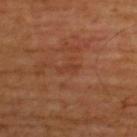Clinical impression:
Imaged during a routine full-body skin examination; the lesion was not biopsied and no histopathology is available.
Image and clinical context:
A male subject aged 63–67. On the upper back. The lesion's longest dimension is about 2.5 mm. Automated image analysis of the tile measured border irregularity of about 4.5 on a 0–10 scale, internal color variation of about 0 on a 0–10 scale, and radial color variation of about 0. The analysis additionally found a detector confidence of about 100 out of 100 that the crop contains a lesion. A lesion tile, about 15 mm wide, cut from a 3D total-body photograph.